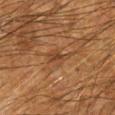follow-up = no biopsy performed (imaged during a skin exam)
automated metrics = a shape eccentricity near 0.8 and a symmetry-axis asymmetry near 0.45; a border-irregularity rating of about 4.5/10 and a color-variation rating of about 2/10
patient = male, aged around 65
site = the left forearm
image = ~15 mm tile from a whole-body skin photo
tile lighting = cross-polarized illumination
diameter = about 3 mm